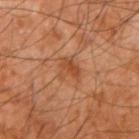{"biopsy_status": "not biopsied; imaged during a skin examination", "image": {"source": "total-body photography crop", "field_of_view_mm": 15}, "lighting": "cross-polarized", "lesion_size": {"long_diameter_mm_approx": 3.0}, "site": "right upper arm", "patient": {"sex": "male", "age_approx": 60}}